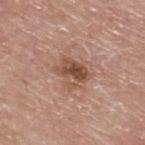The lesion was tiled from a total-body skin photograph and was not biopsied. From the upper back. The subject is a male about 80 years old. Captured under white-light illumination. Longest diameter approximately 3.5 mm. A 15 mm close-up tile from a total-body photography series done for melanoma screening.